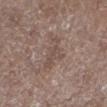{"biopsy_status": "not biopsied; imaged during a skin examination", "lesion_size": {"long_diameter_mm_approx": 4.5}, "patient": {"sex": "male", "age_approx": 45}, "site": "left lower leg", "image": {"source": "total-body photography crop", "field_of_view_mm": 15}, "lighting": "white-light"}